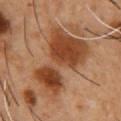The lesion's longest dimension is about 8.5 mm.
The tile uses cross-polarized illumination.
The lesion is on the chest.
The patient is a male aged 53–57.
A 15 mm close-up tile from a total-body photography series done for melanoma screening.
An algorithmic analysis of the crop reported a footprint of about 33 mm² and an eccentricity of roughly 0.8. The analysis additionally found lesion-presence confidence of about 100/100.